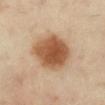Assessment: The lesion was photographed on a routine skin check and not biopsied; there is no pathology result. Background: From the left lower leg. A female subject roughly 40 years of age. The recorded lesion diameter is about 5.5 mm. A lesion tile, about 15 mm wide, cut from a 3D total-body photograph.